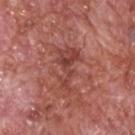follow-up: total-body-photography surveillance lesion; no biopsy | imaging modality: ~15 mm tile from a whole-body skin photo | illumination: white-light illumination | lesion size: about 5.5 mm | automated lesion analysis: an area of roughly 10 mm², an eccentricity of roughly 0.9, and a shape-asymmetry score of about 0.6 (0 = symmetric); a lesion color around L≈44 a*≈28 b*≈26 in CIELAB and a normalized border contrast of about 6.5; an automated nevus-likeness rating near 0 out of 100 and a detector confidence of about 100 out of 100 that the crop contains a lesion | patient: male, aged approximately 60 | location: the head or neck.